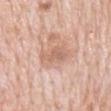No biopsy was performed on this lesion — it was imaged during a full skin examination and was not determined to be concerning. Imaged with white-light lighting. A male subject roughly 80 years of age. The lesion is on the mid back. A 15 mm crop from a total-body photograph taken for skin-cancer surveillance. The recorded lesion diameter is about 3.5 mm. An algorithmic analysis of the crop reported a lesion area of about 6 mm², a shape eccentricity near 0.8, and two-axis asymmetry of about 0.25. It also reported a border-irregularity index near 3/10, a within-lesion color-variation index near 2.5/10, and radial color variation of about 1. The software also gave a nevus-likeness score of about 0/100 and a detector confidence of about 100 out of 100 that the crop contains a lesion.